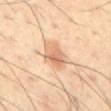biopsy status: imaged on a skin check; not biopsied | anatomic site: the abdomen | patient: male, aged 38 to 42 | lighting: cross-polarized | diameter: ~4 mm (longest diameter) | acquisition: 15 mm crop, total-body photography.